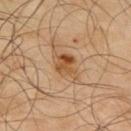automated lesion analysis: a footprint of about 6 mm² and a shape eccentricity near 0.75; a mean CIELAB color near L≈48 a*≈20 b*≈36, roughly 10 lightness units darker than nearby skin, and a normalized border contrast of about 8; a border-irregularity index near 3.5/10, a within-lesion color-variation index near 8/10, and peripheral color asymmetry of about 3; an automated nevus-likeness rating near 55 out of 100 and a detector confidence of about 100 out of 100 that the crop contains a lesion | imaging modality: 15 mm crop, total-body photography | lighting: cross-polarized | subject: male, in their mid-60s | location: the upper back.